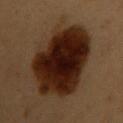{"biopsy_status": "not biopsied; imaged during a skin examination", "image": {"source": "total-body photography crop", "field_of_view_mm": 15}, "automated_metrics": {"area_mm2_approx": 50.0, "eccentricity": 0.7, "shape_asymmetry": 0.2, "border_irregularity_0_10": 2.0, "color_variation_0_10": 7.0, "nevus_likeness_0_100": 100}, "lighting": "cross-polarized", "patient": {"sex": "female", "age_approx": 60}, "site": "back"}